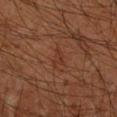The subject is a male aged around 60.
The tile uses cross-polarized illumination.
Cropped from a whole-body photographic skin survey; the tile spans about 15 mm.
Located on the left forearm.